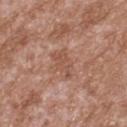follow-up — catalogued during a skin exam; not biopsied | illumination — white-light | body site — the upper back | patient — male, roughly 45 years of age | image source — ~15 mm tile from a whole-body skin photo.